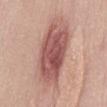Q: Was this lesion biopsied?
A: total-body-photography surveillance lesion; no biopsy